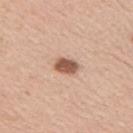notes: total-body-photography surveillance lesion; no biopsy
acquisition: total-body-photography crop, ~15 mm field of view
patient: male, about 30 years old
anatomic site: the arm
tile lighting: white-light illumination
diameter: ≈3 mm
TBP lesion metrics: a footprint of about 5 mm², a shape eccentricity near 0.7, and a shape-asymmetry score of about 0.2 (0 = symmetric); an average lesion color of about L≈56 a*≈21 b*≈29 (CIELAB), about 16 CIELAB-L* units darker than the surrounding skin, and a normalized border contrast of about 10; a border-irregularity index near 1.5/10, internal color variation of about 2.5 on a 0–10 scale, and radial color variation of about 1